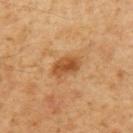Findings:
- workup — total-body-photography surveillance lesion; no biopsy
- tile lighting — cross-polarized illumination
- acquisition — total-body-photography crop, ~15 mm field of view
- location — the mid back
- lesion size — about 3.5 mm
- subject — male, aged approximately 60